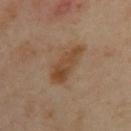Part of a total-body skin-imaging series; this lesion was reviewed on a skin check and was not flagged for biopsy. A female subject, approximately 40 years of age. The tile uses cross-polarized illumination. The lesion-visualizer software estimated a normalized border contrast of about 8. It also reported a nevus-likeness score of about 10/100 and a lesion-detection confidence of about 100/100. Longest diameter approximately 5.5 mm. A close-up tile cropped from a whole-body skin photograph, about 15 mm across. The lesion is located on the arm.